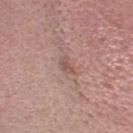Q: Was this lesion biopsied?
A: catalogued during a skin exam; not biopsied
Q: What lighting was used for the tile?
A: white-light illumination
Q: What kind of image is this?
A: 15 mm crop, total-body photography
Q: Patient demographics?
A: female, in their mid-40s
Q: What is the lesion's diameter?
A: about 2.5 mm
Q: Where on the body is the lesion?
A: the head or neck